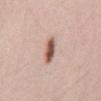Recorded during total-body skin imaging; not selected for excision or biopsy. The lesion is located on the mid back. A 15 mm crop from a total-body photograph taken for skin-cancer surveillance. The patient is a male approximately 35 years of age. This is a white-light tile. An algorithmic analysis of the crop reported a border-irregularity rating of about 2.5/10, a color-variation rating of about 6.5/10, and peripheral color asymmetry of about 2.